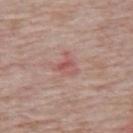Q: How was this image acquired?
A: ~15 mm tile from a whole-body skin photo
Q: Lesion location?
A: the mid back
Q: Patient demographics?
A: male, aged approximately 60